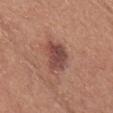notes — no biopsy performed (imaged during a skin exam)
lighting — white-light illumination
lesion size — ~4.5 mm (longest diameter)
automated metrics — a lesion area of about 9.5 mm² and an outline eccentricity of about 0.75 (0 = round, 1 = elongated); an average lesion color of about L≈46 a*≈23 b*≈24 (CIELAB); border irregularity of about 3 on a 0–10 scale, internal color variation of about 4 on a 0–10 scale, and a peripheral color-asymmetry measure near 1
subject — female, aged around 55
location — the chest
image — 15 mm crop, total-body photography